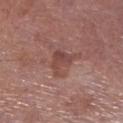{
  "biopsy_status": "not biopsied; imaged during a skin examination",
  "lesion_size": {
    "long_diameter_mm_approx": 3.5
  },
  "site": "right lower leg",
  "patient": {
    "sex": "male",
    "age_approx": 70
  },
  "image": {
    "source": "total-body photography crop",
    "field_of_view_mm": 15
  }
}